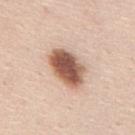Case summary:
- notes — catalogued during a skin exam; not biopsied
- illumination — white-light illumination
- TBP lesion metrics — an area of roughly 13 mm², an eccentricity of roughly 0.75, and two-axis asymmetry of about 0.15; a lesion color around L≈56 a*≈21 b*≈29 in CIELAB; a within-lesion color-variation index near 6.5/10 and radial color variation of about 2
- acquisition — total-body-photography crop, ~15 mm field of view
- anatomic site — the mid back
- subject — male, aged approximately 45
- lesion diameter — about 5 mm